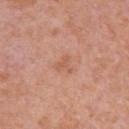{
  "biopsy_status": "not biopsied; imaged during a skin examination",
  "automated_metrics": {
    "eccentricity": 0.5,
    "shape_asymmetry": 0.6,
    "cielab_L": 58,
    "cielab_a": 24,
    "cielab_b": 32,
    "vs_skin_darker_L": 6.0,
    "vs_skin_contrast_norm": 5.0,
    "border_irregularity_0_10": 5.5,
    "color_variation_0_10": 1.5
  },
  "image": {
    "source": "total-body photography crop",
    "field_of_view_mm": 15
  },
  "patient": {
    "sex": "female",
    "age_approx": 40
  },
  "site": "arm",
  "lesion_size": {
    "long_diameter_mm_approx": 2.5
  }
}